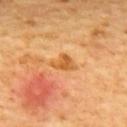* workup: no biopsy performed (imaged during a skin exam)
* size: ≈3 mm
* subject: male, approximately 60 years of age
* location: the upper back
* automated lesion analysis: an area of roughly 4 mm² and an eccentricity of roughly 0.65; lesion-presence confidence of about 100/100
* illumination: cross-polarized illumination
* imaging modality: ~15 mm crop, total-body skin-cancer survey